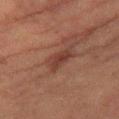Captured during whole-body skin photography for melanoma surveillance; the lesion was not biopsied. The lesion's longest dimension is about 3 mm. A female patient, approximately 55 years of age. This image is a 15 mm lesion crop taken from a total-body photograph. Located on the right lower leg.